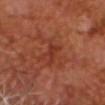No biopsy was performed on this lesion — it was imaged during a full skin examination and was not determined to be concerning.
Approximately 2.5 mm at its widest.
Automated tile analysis of the lesion measured an automated nevus-likeness rating near 0 out of 100 and lesion-presence confidence of about 100/100.
Cropped from a total-body skin-imaging series; the visible field is about 15 mm.
The tile uses cross-polarized illumination.
A male subject, aged 63–67.